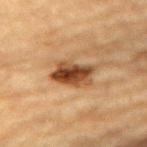Findings:
• biopsy status · imaged on a skin check; not biopsied